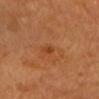This lesion was catalogued during total-body skin photography and was not selected for biopsy.
The lesion is on the head or neck.
The patient is about 70 years old.
A close-up tile cropped from a whole-body skin photograph, about 15 mm across.